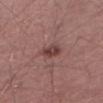follow-up=catalogued during a skin exam; not biopsied
imaging modality=~15 mm tile from a whole-body skin photo
lesion diameter=≈2.5 mm
location=the right thigh
TBP lesion metrics=lesion-presence confidence of about 100/100
tile lighting=white-light
patient=male, about 60 years old